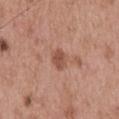The lesion was tiled from a total-body skin photograph and was not biopsied. A male subject aged approximately 55. The tile uses white-light illumination. A 15 mm crop from a total-body photograph taken for skin-cancer surveillance. The lesion is on the mid back.